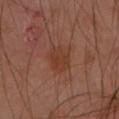Findings:
– follow-up — imaged on a skin check; not biopsied
– patient — female, aged 53–57
– site — the arm
– imaging modality — ~15 mm crop, total-body skin-cancer survey